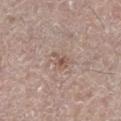Q: Was this lesion biopsied?
A: catalogued during a skin exam; not biopsied
Q: How was this image acquired?
A: ~15 mm crop, total-body skin-cancer survey
Q: What are the patient's age and sex?
A: female, approximately 60 years of age
Q: Where on the body is the lesion?
A: the left lower leg
Q: How large is the lesion?
A: about 2.5 mm